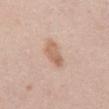Case summary:
• patient · male, aged 48 to 52
• size · ~3.5 mm (longest diameter)
• body site · the back
• image · ~15 mm tile from a whole-body skin photo